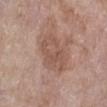This lesion was catalogued during total-body skin photography and was not selected for biopsy.
Imaged with white-light lighting.
On the left lower leg.
A female subject in their mid-60s.
A 15 mm crop from a total-body photograph taken for skin-cancer surveillance.
Approximately 4.5 mm at its widest.